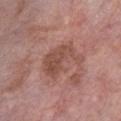<case>
<biopsy_status>not biopsied; imaged during a skin examination</biopsy_status>
<lesion_size>
  <long_diameter_mm_approx>5.5</long_diameter_mm_approx>
</lesion_size>
<lighting>white-light</lighting>
<site>chest</site>
<image>
  <source>total-body photography crop</source>
  <field_of_view_mm>15</field_of_view_mm>
</image>
<patient>
  <sex>male</sex>
  <age_approx>60</age_approx>
</patient>
</case>